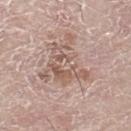<record>
<biopsy_status>not biopsied; imaged during a skin examination</biopsy_status>
<site>left leg</site>
<patient>
  <sex>male</sex>
  <age_approx>80</age_approx>
</patient>
<lighting>white-light</lighting>
<image>
  <source>total-body photography crop</source>
  <field_of_view_mm>15</field_of_view_mm>
</image>
<lesion_size>
  <long_diameter_mm_approx>5.5</long_diameter_mm_approx>
</lesion_size>
</record>